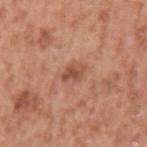• notes — total-body-photography surveillance lesion; no biopsy
• location — the arm
• image source — 15 mm crop, total-body photography
• lighting — white-light illumination
• subject — male, about 50 years old
• image-analysis metrics — internal color variation of about 3.5 on a 0–10 scale and radial color variation of about 1; an automated nevus-likeness rating near 5 out of 100 and lesion-presence confidence of about 100/100